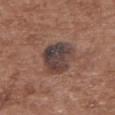Assessment: Part of a total-body skin-imaging series; this lesion was reviewed on a skin check and was not flagged for biopsy. Context: Measured at roughly 4.5 mm in maximum diameter. The patient is a female approximately 75 years of age. An algorithmic analysis of the crop reported a lesion color around L≈40 a*≈15 b*≈19 in CIELAB, a lesion–skin lightness drop of about 12, and a normalized border contrast of about 11. And it measured a border-irregularity rating of about 2/10, a within-lesion color-variation index near 7.5/10, and radial color variation of about 2.5. The lesion is located on the upper back. This is a white-light tile. A 15 mm crop from a total-body photograph taken for skin-cancer surveillance.